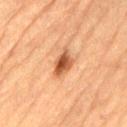<record>
  <biopsy_status>not biopsied; imaged during a skin examination</biopsy_status>
  <image>
    <source>total-body photography crop</source>
    <field_of_view_mm>15</field_of_view_mm>
  </image>
  <site>lower back</site>
  <lighting>cross-polarized</lighting>
  <lesion_size>
    <long_diameter_mm_approx>3.5</long_diameter_mm_approx>
  </lesion_size>
  <patient>
    <sex>male</sex>
    <age_approx>85+</age_approx>
  </patient>
</record>